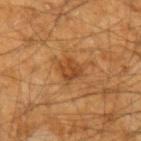Impression: Imaged during a routine full-body skin examination; the lesion was not biopsied and no histopathology is available. Acquisition and patient details: A male patient, approximately 60 years of age. A roughly 15 mm field-of-view crop from a total-body skin photograph. From the left forearm.